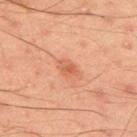Captured during whole-body skin photography for melanoma surveillance; the lesion was not biopsied. A male patient, in their mid- to late 40s. Located on the upper back. This image is a 15 mm lesion crop taken from a total-body photograph. The recorded lesion diameter is about 2.5 mm. This is a cross-polarized tile.